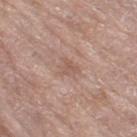Image and clinical context: A 15 mm close-up tile from a total-body photography series done for melanoma screening. A female subject, aged 63 to 67. Automated tile analysis of the lesion measured a border-irregularity rating of about 3/10, a within-lesion color-variation index near 1.5/10, and a peripheral color-asymmetry measure near 0.5. Imaged with white-light lighting. From the right thigh.